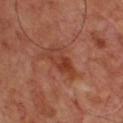<tbp_lesion>
<biopsy_status>not biopsied; imaged during a skin examination</biopsy_status>
<patient>
  <sex>male</sex>
  <age_approx>65</age_approx>
</patient>
<image>
  <source>total-body photography crop</source>
  <field_of_view_mm>15</field_of_view_mm>
</image>
<lesion_size>
  <long_diameter_mm_approx>4.5</long_diameter_mm_approx>
</lesion_size>
<lighting>cross-polarized</lighting>
</tbp_lesion>